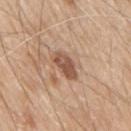Recorded during total-body skin imaging; not selected for excision or biopsy. Automated image analysis of the tile measured a lesion-to-skin contrast of about 8.5 (normalized; higher = more distinct). The analysis additionally found a color-variation rating of about 4.5/10 and a peripheral color-asymmetry measure near 1.5. The software also gave a detector confidence of about 100 out of 100 that the crop contains a lesion. The subject is a male approximately 80 years of age. The lesion is located on the mid back. Approximately 3.5 mm at its widest. A 15 mm close-up tile from a total-body photography series done for melanoma screening.